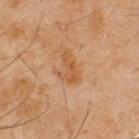Captured during whole-body skin photography for melanoma surveillance; the lesion was not biopsied. On the upper back. A 15 mm close-up extracted from a 3D total-body photography capture. The patient is a male aged 43 to 47. This is a cross-polarized tile. The recorded lesion diameter is about 3.5 mm.